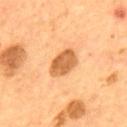Clinical impression: Imaged during a routine full-body skin examination; the lesion was not biopsied and no histopathology is available. Background: This is a cross-polarized tile. A 15 mm close-up tile from a total-body photography series done for melanoma screening. From the upper back. A male patient about 55 years old.